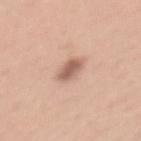No biopsy was performed on this lesion — it was imaged during a full skin examination and was not determined to be concerning. A 15 mm crop from a total-body photograph taken for skin-cancer surveillance. Imaged with white-light lighting. Located on the abdomen. Automated tile analysis of the lesion measured border irregularity of about 2.5 on a 0–10 scale and a peripheral color-asymmetry measure near 1. The software also gave an automated nevus-likeness rating near 100 out of 100 and a lesion-detection confidence of about 100/100. The lesion's longest dimension is about 4 mm. A female subject, aged approximately 35.